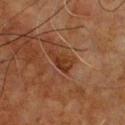• follow-up · total-body-photography surveillance lesion; no biopsy
• patient · male, roughly 60 years of age
• image · 15 mm crop, total-body photography
• location · the chest